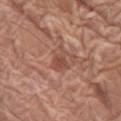Q: Was a biopsy performed?
A: no biopsy performed (imaged during a skin exam)
Q: Who is the patient?
A: female, aged 73–77
Q: Where on the body is the lesion?
A: the left thigh
Q: How was this image acquired?
A: ~15 mm tile from a whole-body skin photo
Q: How was the tile lit?
A: white-light
Q: Automated lesion metrics?
A: an area of roughly 5 mm², a shape eccentricity near 0.7, and a symmetry-axis asymmetry near 0.45; border irregularity of about 5 on a 0–10 scale and peripheral color asymmetry of about 1; an automated nevus-likeness rating near 0 out of 100 and lesion-presence confidence of about 100/100
Q: What is the lesion's diameter?
A: about 3 mm